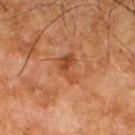No biopsy was performed on this lesion — it was imaged during a full skin examination and was not determined to be concerning. The patient is a male aged approximately 80. The recorded lesion diameter is about 3.5 mm. The tile uses cross-polarized illumination. Cropped from a total-body skin-imaging series; the visible field is about 15 mm. Located on the right thigh.